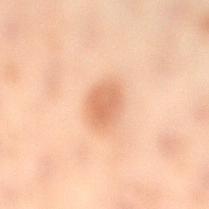The lesion was photographed on a routine skin check and not biopsied; there is no pathology result. A close-up tile cropped from a whole-body skin photograph, about 15 mm across. Located on the left leg. The lesion-visualizer software estimated an average lesion color of about L≈57 a*≈21 b*≈32 (CIELAB) and a normalized lesion–skin contrast near 6.5. It also reported a nevus-likeness score of about 90/100 and lesion-presence confidence of about 100/100. The tile uses cross-polarized illumination. Approximately 3.5 mm at its widest. The subject is a female approximately 55 years of age.